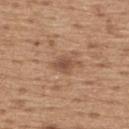The lesion was tiled from a total-body skin photograph and was not biopsied. Automated tile analysis of the lesion measured a lesion area of about 3.5 mm² and two-axis asymmetry of about 0.2. It also reported a border-irregularity index near 2/10, a color-variation rating of about 1.5/10, and peripheral color asymmetry of about 0.5. And it measured a classifier nevus-likeness of about 15/100. On the upper back. A 15 mm close-up extracted from a 3D total-body photography capture. About 2.5 mm across. The patient is a male aged 63 to 67.